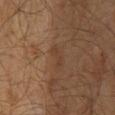biopsy status = catalogued during a skin exam; not biopsied | image source = ~15 mm crop, total-body skin-cancer survey | size = ~3 mm (longest diameter) | subject = male, aged 58 to 62 | anatomic site = the mid back | tile lighting = cross-polarized illumination.